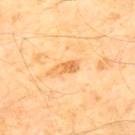The lesion was tiled from a total-body skin photograph and was not biopsied. An algorithmic analysis of the crop reported a lesion area of about 3.5 mm², an eccentricity of roughly 0.9, and a symmetry-axis asymmetry near 0.25. The software also gave a mean CIELAB color near L≈70 a*≈25 b*≈49, a lesion–skin lightness drop of about 10, and a lesion-to-skin contrast of about 7 (normalized; higher = more distinct). The software also gave a nevus-likeness score of about 0/100 and a lesion-detection confidence of about 100/100. The recorded lesion diameter is about 3 mm. This is a cross-polarized tile. The subject is a male aged 53 to 57. On the upper back. A region of skin cropped from a whole-body photographic capture, roughly 15 mm wide.